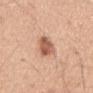Imaged during a routine full-body skin examination; the lesion was not biopsied and no histopathology is available.
Captured under white-light illumination.
Automated image analysis of the tile measured a lesion area of about 7 mm², an eccentricity of roughly 0.85, and a shape-asymmetry score of about 0.25 (0 = symmetric). The analysis additionally found an average lesion color of about L≈58 a*≈24 b*≈31 (CIELAB), about 14 CIELAB-L* units darker than the surrounding skin, and a lesion-to-skin contrast of about 9 (normalized; higher = more distinct).
The lesion's longest dimension is about 4.5 mm.
On the mid back.
The patient is a male in their mid-40s.
Cropped from a total-body skin-imaging series; the visible field is about 15 mm.